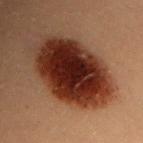Clinical impression: Captured during whole-body skin photography for melanoma surveillance; the lesion was not biopsied. Context: On the chest. A female patient roughly 20 years of age. Automated image analysis of the tile measured a lesion area of about 40 mm², a shape eccentricity near 0.8, and two-axis asymmetry of about 0.1. And it measured a border-irregularity rating of about 1.5/10 and a peripheral color-asymmetry measure near 1.5. The software also gave a nevus-likeness score of about 100/100 and a detector confidence of about 100 out of 100 that the crop contains a lesion. Approximately 9 mm at its widest. A close-up tile cropped from a whole-body skin photograph, about 15 mm across.